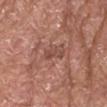The patient is a male aged 63 to 67. Cropped from a whole-body photographic skin survey; the tile spans about 15 mm. Captured under white-light illumination. Longest diameter approximately 3 mm. The lesion is located on the head or neck. Automated image analysis of the tile measured an average lesion color of about L≈48 a*≈23 b*≈26 (CIELAB), a lesion–skin lightness drop of about 7, and a normalized lesion–skin contrast near 5. The analysis additionally found a nevus-likeness score of about 0/100 and a detector confidence of about 100 out of 100 that the crop contains a lesion.